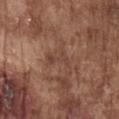notes: catalogued during a skin exam; not biopsied
size: ~4 mm (longest diameter)
patient: male, aged approximately 75
tile lighting: white-light
TBP lesion metrics: an area of roughly 6.5 mm², an outline eccentricity of about 0.75 (0 = round, 1 = elongated), and a shape-asymmetry score of about 0.7 (0 = symmetric); a lesion color around L≈43 a*≈19 b*≈26 in CIELAB, a lesion–skin lightness drop of about 6, and a normalized border contrast of about 4.5; a border-irregularity rating of about 8.5/10 and internal color variation of about 2 on a 0–10 scale; an automated nevus-likeness rating near 0 out of 100
acquisition: ~15 mm tile from a whole-body skin photo
site: the chest